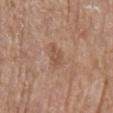Recorded during total-body skin imaging; not selected for excision or biopsy. From the left upper arm. A roughly 15 mm field-of-view crop from a total-body skin photograph. Longest diameter approximately 3 mm. A female subject, aged 83 or over. Imaged with white-light lighting.